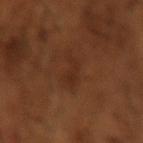Clinical impression:
Captured during whole-body skin photography for melanoma surveillance; the lesion was not biopsied.
Acquisition and patient details:
About 3 mm across. Cropped from a total-body skin-imaging series; the visible field is about 15 mm. The lesion is on the right forearm. The subject is a male roughly 65 years of age. The tile uses cross-polarized illumination.